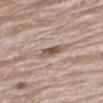Findings:
• automated lesion analysis: an area of roughly 5 mm², an outline eccentricity of about 0.9 (0 = round, 1 = elongated), and a shape-asymmetry score of about 0.25 (0 = symmetric); a border-irregularity rating of about 3/10; an automated nevus-likeness rating near 40 out of 100 and lesion-presence confidence of about 100/100
• image: ~15 mm tile from a whole-body skin photo
• lesion size: about 4 mm
• patient: male, aged approximately 65
• location: the right thigh